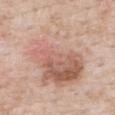Captured during whole-body skin photography for melanoma surveillance; the lesion was not biopsied. A region of skin cropped from a whole-body photographic capture, roughly 15 mm wide. The lesion is on the upper back. A male subject, approximately 50 years of age. The lesion's longest dimension is about 10.5 mm. Imaged with white-light lighting.